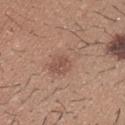This lesion was catalogued during total-body skin photography and was not selected for biopsy. A 15 mm close-up tile from a total-body photography series done for melanoma screening. Captured under white-light illumination. About 5 mm across. A male patient roughly 25 years of age. From the upper back. Automated tile analysis of the lesion measured border irregularity of about 3.5 on a 0–10 scale, a color-variation rating of about 4.5/10, and radial color variation of about 1.5. The software also gave a nevus-likeness score of about 35/100 and a lesion-detection confidence of about 100/100.